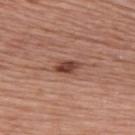Assessment:
No biopsy was performed on this lesion — it was imaged during a full skin examination and was not determined to be concerning.
Acquisition and patient details:
A female patient in their mid- to late 60s. Located on the upper back. This is a white-light tile. The lesion-visualizer software estimated a footprint of about 4.5 mm², an outline eccentricity of about 0.85 (0 = round, 1 = elongated), and two-axis asymmetry of about 0.2. And it measured a border-irregularity index near 2/10, a within-lesion color-variation index near 3.5/10, and peripheral color asymmetry of about 1.5. A close-up tile cropped from a whole-body skin photograph, about 15 mm across. The lesion's longest dimension is about 3 mm.